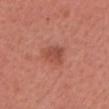workup: total-body-photography surveillance lesion; no biopsy | lighting: white-light | lesion size: ~3.5 mm (longest diameter) | body site: the head or neck | image: ~15 mm tile from a whole-body skin photo | subject: female, about 65 years old.